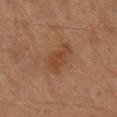workup: total-body-photography surveillance lesion; no biopsy | lesion diameter: about 4 mm | site: the right lower leg | image: total-body-photography crop, ~15 mm field of view | lighting: cross-polarized | subject: male, in their mid-80s or older.